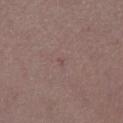Clinical impression:
Recorded during total-body skin imaging; not selected for excision or biopsy.
Acquisition and patient details:
A female patient, in their 30s. The lesion is on the right lower leg. An algorithmic analysis of the crop reported a border-irregularity rating of about 3.5/10 and peripheral color asymmetry of about 0. A roughly 15 mm field-of-view crop from a total-body skin photograph. Imaged with white-light lighting. About 1 mm across.